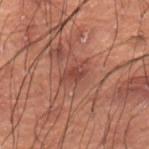<tbp_lesion>
  <biopsy_status>not biopsied; imaged during a skin examination</biopsy_status>
  <lesion_size>
    <long_diameter_mm_approx>3.0</long_diameter_mm_approx>
  </lesion_size>
  <automated_metrics>
    <area_mm2_approx>4.0</area_mm2_approx>
    <eccentricity>0.85</eccentricity>
    <shape_asymmetry>0.25</shape_asymmetry>
    <peripheral_color_asymmetry>1.0</peripheral_color_asymmetry>
    <lesion_detection_confidence_0_100>100</lesion_detection_confidence_0_100>
  </automated_metrics>
  <patient>
    <sex>male</sex>
    <age_approx>50</age_approx>
  </patient>
  <image>
    <source>total-body photography crop</source>
    <field_of_view_mm>15</field_of_view_mm>
  </image>
  <lighting>cross-polarized</lighting>
  <site>lower back</site>
</tbp_lesion>